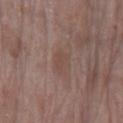| key | value |
|---|---|
| workup | catalogued during a skin exam; not biopsied |
| subject | female, aged 68 to 72 |
| tile lighting | white-light |
| location | the left lower leg |
| lesion diameter | about 3 mm |
| imaging modality | 15 mm crop, total-body photography |